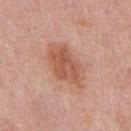The patient is a male in their mid- to late 50s. The lesion's longest dimension is about 6.5 mm. Captured under white-light illumination. The lesion is located on the chest. A lesion tile, about 15 mm wide, cut from a 3D total-body photograph.